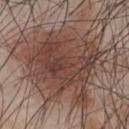biopsy status — imaged on a skin check; not biopsied | size — ≈8.5 mm | imaging modality — 15 mm crop, total-body photography | body site — the chest | subject — male, aged approximately 45 | illumination — white-light illumination.